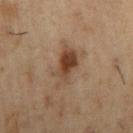Q: Was this lesion biopsied?
A: total-body-photography surveillance lesion; no biopsy
Q: What kind of image is this?
A: ~15 mm crop, total-body skin-cancer survey
Q: Automated lesion metrics?
A: a mean CIELAB color near L≈42 a*≈18 b*≈29, a lesion–skin lightness drop of about 11, and a lesion-to-skin contrast of about 9 (normalized; higher = more distinct); a nevus-likeness score of about 90/100 and a lesion-detection confidence of about 100/100
Q: Where on the body is the lesion?
A: the right upper arm
Q: What is the lesion's diameter?
A: ≈4.5 mm
Q: Illumination type?
A: cross-polarized illumination
Q: Patient demographics?
A: male, aged 53–57